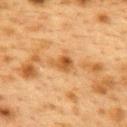Impression:
No biopsy was performed on this lesion — it was imaged during a full skin examination and was not determined to be concerning.
Context:
The patient is a female aged 38 to 42. Approximately 2.5 mm at its widest. A roughly 15 mm field-of-view crop from a total-body skin photograph. Located on the upper back. Captured under cross-polarized illumination.